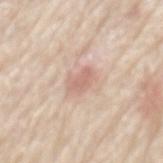Impression:
The lesion was photographed on a routine skin check and not biopsied; there is no pathology result.
Clinical summary:
Measured at roughly 3 mm in maximum diameter. A male patient aged around 80. Located on the mid back. A 15 mm close-up extracted from a 3D total-body photography capture.